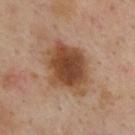Case summary:
• notes — catalogued during a skin exam; not biopsied
• lesion diameter — ≈6.5 mm
• image-analysis metrics — a color-variation rating of about 5/10 and peripheral color asymmetry of about 1.5
• tile lighting — cross-polarized
• subject — male, aged 43–47
• image — ~15 mm tile from a whole-body skin photo
• body site — the upper back